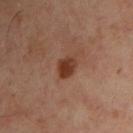The subject is a male roughly 50 years of age. This is a cross-polarized tile. The recorded lesion diameter is about 3 mm. Located on the chest. This image is a 15 mm lesion crop taken from a total-body photograph.